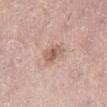{"biopsy_status": "not biopsied; imaged during a skin examination", "image": {"source": "total-body photography crop", "field_of_view_mm": 15}, "lighting": "white-light", "patient": {"sex": "female", "age_approx": 40}, "site": "left lower leg", "automated_metrics": {"area_mm2_approx": 5.0, "eccentricity": 0.85, "shape_asymmetry": 0.25, "vs_skin_darker_L": 11.0, "vs_skin_contrast_norm": 7.0, "nevus_likeness_0_100": 25, "lesion_detection_confidence_0_100": 100}, "lesion_size": {"long_diameter_mm_approx": 3.5}}